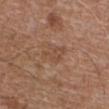Captured under white-light illumination.
The lesion is located on the abdomen.
A male patient about 75 years old.
The lesion-visualizer software estimated a mean CIELAB color near L≈47 a*≈20 b*≈30, roughly 6 lightness units darker than nearby skin, and a normalized border contrast of about 5. The software also gave a nevus-likeness score of about 0/100.
Longest diameter approximately 3.5 mm.
A close-up tile cropped from a whole-body skin photograph, about 15 mm across.